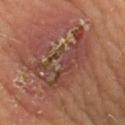Q: Was this lesion biopsied?
A: catalogued during a skin exam; not biopsied
Q: Where on the body is the lesion?
A: the left thigh
Q: Illumination type?
A: cross-polarized
Q: How was this image acquired?
A: ~15 mm crop, total-body skin-cancer survey
Q: Lesion size?
A: ≈8 mm
Q: Patient demographics?
A: male, aged 38–42
Q: Automated lesion metrics?
A: a footprint of about 24 mm² and an eccentricity of roughly 0.85; a mean CIELAB color near L≈42 a*≈21 b*≈26; a border-irregularity rating of about 6/10, a within-lesion color-variation index near 9/10, and radial color variation of about 3